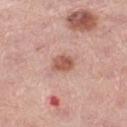Q: Is there a histopathology result?
A: catalogued during a skin exam; not biopsied
Q: What kind of image is this?
A: ~15 mm tile from a whole-body skin photo
Q: How large is the lesion?
A: ≈2.5 mm
Q: What are the patient's age and sex?
A: female, about 45 years old
Q: What is the anatomic site?
A: the left thigh